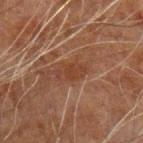notes = catalogued during a skin exam; not biopsied
patient = male, aged around 60
anatomic site = the left forearm
imaging modality = ~15 mm tile from a whole-body skin photo
diameter = ~4 mm (longest diameter)